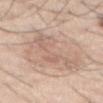Captured during whole-body skin photography for melanoma surveillance; the lesion was not biopsied. A male patient aged around 45. The lesion is located on the abdomen. An algorithmic analysis of the crop reported an eccentricity of roughly 0.95 and two-axis asymmetry of about 0.65. And it measured a border-irregularity rating of about 9.5/10 and peripheral color asymmetry of about 1. Captured under white-light illumination. A roughly 15 mm field-of-view crop from a total-body skin photograph.